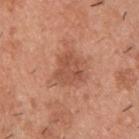The lesion was photographed on a routine skin check and not biopsied; there is no pathology result.
Cropped from a whole-body photographic skin survey; the tile spans about 15 mm.
A male patient, approximately 40 years of age.
Measured at roughly 3.5 mm in maximum diameter.
The lesion is on the upper back.
Automated tile analysis of the lesion measured a classifier nevus-likeness of about 5/100.